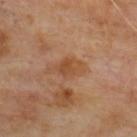The lesion was tiled from a total-body skin photograph and was not biopsied.
This is a cross-polarized tile.
Automated tile analysis of the lesion measured a lesion color around L≈46 a*≈21 b*≈34 in CIELAB, about 7 CIELAB-L* units darker than the surrounding skin, and a lesion-to-skin contrast of about 6 (normalized; higher = more distinct). The analysis additionally found an automated nevus-likeness rating near 0 out of 100.
A male patient, aged 63 to 67.
A lesion tile, about 15 mm wide, cut from a 3D total-body photograph.
On the upper back.